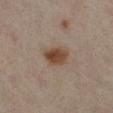| feature | finding |
|---|---|
| notes | no biopsy performed (imaged during a skin exam) |
| patient | female, about 45 years old |
| anatomic site | the left lower leg |
| TBP lesion metrics | an outline eccentricity of about 0.7 (0 = round, 1 = elongated) and two-axis asymmetry of about 0.25; a lesion color around L≈37 a*≈14 b*≈24 in CIELAB, a lesion–skin lightness drop of about 10, and a normalized lesion–skin contrast near 9.5; border irregularity of about 2 on a 0–10 scale, a color-variation rating of about 4/10, and radial color variation of about 1.5; lesion-presence confidence of about 100/100 |
| imaging modality | ~15 mm crop, total-body skin-cancer survey |
| size | ≈3.5 mm |
| tile lighting | cross-polarized |